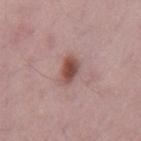Findings:
• follow-up: imaged on a skin check; not biopsied
• site: the mid back
• image: ~15 mm crop, total-body skin-cancer survey
• image-analysis metrics: a footprint of about 5 mm² and a shape eccentricity near 0.7; a lesion color around L≈49 a*≈22 b*≈23 in CIELAB, a lesion–skin lightness drop of about 13, and a lesion-to-skin contrast of about 9.5 (normalized; higher = more distinct); a border-irregularity rating of about 2/10 and a peripheral color-asymmetry measure near 1.5; a classifier nevus-likeness of about 95/100 and lesion-presence confidence of about 100/100
• patient: male, about 70 years old
• lighting: white-light